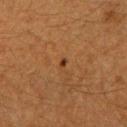- subject — female, approximately 40 years of age
- anatomic site — the right forearm
- image — ~15 mm tile from a whole-body skin photo